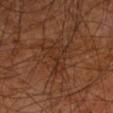follow-up: no biopsy performed (imaged during a skin exam); patient: male, aged approximately 60; site: the arm; imaging modality: ~15 mm tile from a whole-body skin photo.